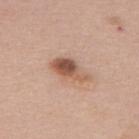Recorded during total-body skin imaging; not selected for excision or biopsy. The recorded lesion diameter is about 5 mm. A female subject, aged 58 to 62. The lesion is located on the upper back. Cropped from a whole-body photographic skin survey; the tile spans about 15 mm. Captured under white-light illumination.